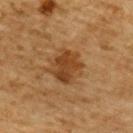Assessment: Imaged during a routine full-body skin examination; the lesion was not biopsied and no histopathology is available. Acquisition and patient details: The lesion-visualizer software estimated a lesion area of about 12 mm², an outline eccentricity of about 0.45 (0 = round, 1 = elongated), and a symmetry-axis asymmetry near 0.2. The software also gave a border-irregularity rating of about 2.5/10 and a color-variation rating of about 4/10. The recorded lesion diameter is about 4 mm. A 15 mm close-up extracted from a 3D total-body photography capture. This is a cross-polarized tile. A male patient aged 83 or older. Located on the back.